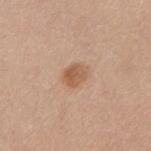  biopsy_status: not biopsied; imaged during a skin examination
  patient:
    sex: female
    age_approx: 40
  site: right forearm
  image:
    source: total-body photography crop
    field_of_view_mm: 15
  automated_metrics:
    vs_skin_contrast_norm: 7.5
    nevus_likeness_0_100: 90
    lesion_detection_confidence_0_100: 100